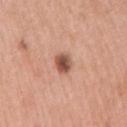follow-up: total-body-photography surveillance lesion; no biopsy
patient: male, about 60 years old
illumination: white-light illumination
site: the arm
image-analysis metrics: an area of roughly 4.5 mm² and two-axis asymmetry of about 0.15; an average lesion color of about L≈53 a*≈24 b*≈29 (CIELAB), a lesion–skin lightness drop of about 15, and a normalized lesion–skin contrast near 10; a border-irregularity index near 1.5/10 and a peripheral color-asymmetry measure near 1.5; a classifier nevus-likeness of about 95/100 and lesion-presence confidence of about 100/100
image: ~15 mm crop, total-body skin-cancer survey